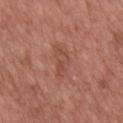Q: Was a biopsy performed?
A: total-body-photography surveillance lesion; no biopsy
Q: Illumination type?
A: white-light illumination
Q: How large is the lesion?
A: about 4.5 mm
Q: What did automated image analysis measure?
A: a peripheral color-asymmetry measure near 1
Q: How was this image acquired?
A: ~15 mm tile from a whole-body skin photo
Q: Lesion location?
A: the back
Q: Patient demographics?
A: male, aged approximately 50